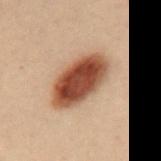Q: Was a biopsy performed?
A: total-body-photography surveillance lesion; no biopsy